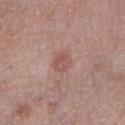Part of a total-body skin-imaging series; this lesion was reviewed on a skin check and was not flagged for biopsy.
The lesion is located on the right lower leg.
This is a white-light tile.
A close-up tile cropped from a whole-body skin photograph, about 15 mm across.
A male patient, aged 48–52.